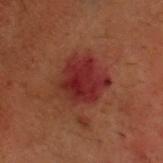Q: Was this lesion biopsied?
A: imaged on a skin check; not biopsied
Q: Where on the body is the lesion?
A: the head or neck
Q: What kind of image is this?
A: ~15 mm tile from a whole-body skin photo
Q: Automated lesion metrics?
A: roughly 7 lightness units darker than nearby skin and a normalized lesion–skin contrast near 9; a border-irregularity index near 2/10 and a within-lesion color-variation index near 5/10
Q: What are the patient's age and sex?
A: male, aged 48 to 52